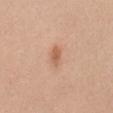  biopsy_status: not biopsied; imaged during a skin examination
  lighting: white-light
  site: mid back
  patient:
    sex: female
    age_approx: 50
  image:
    source: total-body photography crop
    field_of_view_mm: 15
  lesion_size:
    long_diameter_mm_approx: 2.5